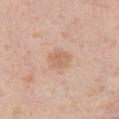Q: Was this lesion biopsied?
A: no biopsy performed (imaged during a skin exam)
Q: What is the lesion's diameter?
A: about 3 mm
Q: What is the anatomic site?
A: the left thigh
Q: Patient demographics?
A: female, aged around 40
Q: How was the tile lit?
A: white-light
Q: How was this image acquired?
A: 15 mm crop, total-body photography